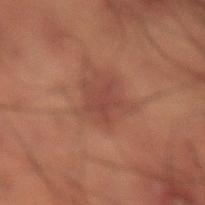Assessment:
Imaged during a routine full-body skin examination; the lesion was not biopsied and no histopathology is available.
Image and clinical context:
A 15 mm close-up extracted from a 3D total-body photography capture. On the lower back. A male patient in their 50s. Captured under cross-polarized illumination. The lesion's longest dimension is about 3 mm.